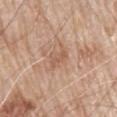Imaged during a routine full-body skin examination; the lesion was not biopsied and no histopathology is available.